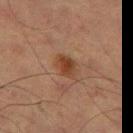Assessment:
This lesion was catalogued during total-body skin photography and was not selected for biopsy.
Acquisition and patient details:
Approximately 3.5 mm at its widest. Automated tile analysis of the lesion measured a lesion area of about 5.5 mm² and an outline eccentricity of about 0.75 (0 = round, 1 = elongated). The analysis additionally found an average lesion color of about L≈33 a*≈18 b*≈26 (CIELAB), about 8 CIELAB-L* units darker than the surrounding skin, and a normalized border contrast of about 8.5. And it measured internal color variation of about 3 on a 0–10 scale and peripheral color asymmetry of about 1. A male patient about 65 years old. The tile uses cross-polarized illumination. On the right thigh. A 15 mm close-up extracted from a 3D total-body photography capture.